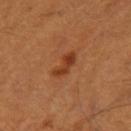follow-up = catalogued during a skin exam; not biopsied | anatomic site = the right thigh | acquisition = 15 mm crop, total-body photography | subject = male, roughly 55 years of age.